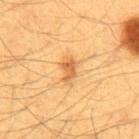Assessment: This lesion was catalogued during total-body skin photography and was not selected for biopsy. Acquisition and patient details: Captured under cross-polarized illumination. From the abdomen. The total-body-photography lesion software estimated a lesion–skin lightness drop of about 11 and a normalized lesion–skin contrast near 7. The software also gave a border-irregularity rating of about 3/10 and internal color variation of about 2.5 on a 0–10 scale. The analysis additionally found an automated nevus-likeness rating near 65 out of 100 and lesion-presence confidence of about 100/100. A male patient aged around 60. A 15 mm close-up extracted from a 3D total-body photography capture. Approximately 3 mm at its widest.